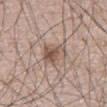No biopsy was performed on this lesion — it was imaged during a full skin examination and was not determined to be concerning.
Cropped from a whole-body photographic skin survey; the tile spans about 15 mm.
Imaged with white-light lighting.
From the front of the torso.
The subject is a male aged 48–52.
The lesion's longest dimension is about 4 mm.